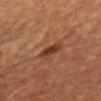<case>
<biopsy_status>not biopsied; imaged during a skin examination</biopsy_status>
<site>chest</site>
<automated_metrics>
  <cielab_L>31</cielab_L>
  <cielab_a>21</cielab_a>
  <cielab_b>28</cielab_b>
  <vs_skin_contrast_norm>8.5</vs_skin_contrast_norm>
  <border_irregularity_0_10>2.5</border_irregularity_0_10>
  <color_variation_0_10>2.0</color_variation_0_10>
  <peripheral_color_asymmetry>0.5</peripheral_color_asymmetry>
  <nevus_likeness_0_100>80</nevus_likeness_0_100>
  <lesion_detection_confidence_0_100>100</lesion_detection_confidence_0_100>
</automated_metrics>
<lesion_size>
  <long_diameter_mm_approx>3.0</long_diameter_mm_approx>
</lesion_size>
<image>
  <source>total-body photography crop</source>
  <field_of_view_mm>15</field_of_view_mm>
</image>
<patient>
  <sex>female</sex>
  <age_approx>50</age_approx>
</patient>
<lighting>cross-polarized</lighting>
</case>